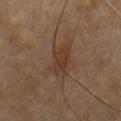A 15 mm close-up tile from a total-body photography series done for melanoma screening. This is a cross-polarized tile. Approximately 4 mm at its widest. A male subject, roughly 60 years of age. Located on the upper back.